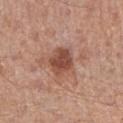Captured during whole-body skin photography for melanoma surveillance; the lesion was not biopsied. The lesion is located on the right forearm. Automated image analysis of the tile measured border irregularity of about 2 on a 0–10 scale and radial color variation of about 1. The subject is a female aged approximately 50. The lesion's longest dimension is about 3.5 mm. A roughly 15 mm field-of-view crop from a total-body skin photograph.